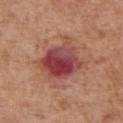Imaged during a routine full-body skin examination; the lesion was not biopsied and no histopathology is available.
A female patient, aged approximately 75.
Measured at roughly 5 mm in maximum diameter.
A 15 mm close-up extracted from a 3D total-body photography capture.
Captured under white-light illumination.
On the chest.